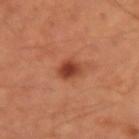| feature | finding |
|---|---|
| workup | catalogued during a skin exam; not biopsied |
| acquisition | ~15 mm tile from a whole-body skin photo |
| subject | male, in their mid- to late 50s |
| body site | the left upper arm |
| image-analysis metrics | a lesion color around L≈41 a*≈29 b*≈33 in CIELAB, roughly 12 lightness units darker than nearby skin, and a normalized border contrast of about 9.5; border irregularity of about 2.5 on a 0–10 scale and peripheral color asymmetry of about 1 |
| lighting | cross-polarized illumination |